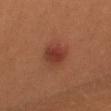This lesion was catalogued during total-body skin photography and was not selected for biopsy.
A female subject, aged 28–32.
On the left leg.
A 15 mm close-up tile from a total-body photography series done for melanoma screening.
Approximately 3 mm at its widest.